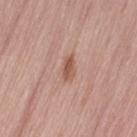Q: Was a biopsy performed?
A: imaged on a skin check; not biopsied
Q: What are the patient's age and sex?
A: male, roughly 55 years of age
Q: Illumination type?
A: white-light
Q: Lesion location?
A: the lower back
Q: Lesion size?
A: about 3 mm
Q: What is the imaging modality?
A: ~15 mm tile from a whole-body skin photo